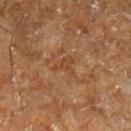Q: Was a biopsy performed?
A: catalogued during a skin exam; not biopsied
Q: What is the imaging modality?
A: ~15 mm tile from a whole-body skin photo
Q: Lesion size?
A: ~3 mm (longest diameter)
Q: Automated lesion metrics?
A: an average lesion color of about L≈33 a*≈17 b*≈28 (CIELAB) and roughly 5 lightness units darker than nearby skin; a border-irregularity index near 8/10, a color-variation rating of about 1/10, and a peripheral color-asymmetry measure near 0.5
Q: Patient demographics?
A: male, aged around 60
Q: What is the anatomic site?
A: the right lower leg